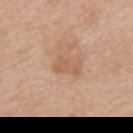Impression: The lesion was tiled from a total-body skin photograph and was not biopsied. Context: Cropped from a total-body skin-imaging series; the visible field is about 15 mm. Located on the mid back. The subject is a female aged 63 to 67. The tile uses white-light illumination. An algorithmic analysis of the crop reported a shape eccentricity near 0.8 and a shape-asymmetry score of about 0.4 (0 = symmetric). The analysis additionally found a border-irregularity rating of about 4.5/10, a color-variation rating of about 1/10, and peripheral color asymmetry of about 0.5.